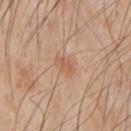Part of a total-body skin-imaging series; this lesion was reviewed on a skin check and was not flagged for biopsy. The subject is a male aged 63–67. About 2.5 mm across. A region of skin cropped from a whole-body photographic capture, roughly 15 mm wide. Imaged with white-light lighting. On the right upper arm.